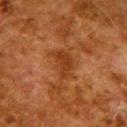<tbp_lesion>
<biopsy_status>not biopsied; imaged during a skin examination</biopsy_status>
<patient>
  <sex>female</sex>
  <age_approx>50</age_approx>
</patient>
<site>upper back</site>
<image>
  <source>total-body photography crop</source>
  <field_of_view_mm>15</field_of_view_mm>
</image>
</tbp_lesion>